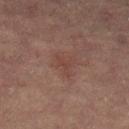Captured during whole-body skin photography for melanoma surveillance; the lesion was not biopsied. The lesion-visualizer software estimated a lesion area of about 3 mm² and a symmetry-axis asymmetry near 0.5. The lesion's longest dimension is about 2.5 mm. The subject is a female in their 70s. A 15 mm crop from a total-body photograph taken for skin-cancer surveillance. The lesion is on the leg. Imaged with cross-polarized lighting.